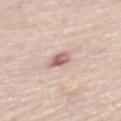Captured during whole-body skin photography for melanoma surveillance; the lesion was not biopsied. A 15 mm crop from a total-body photograph taken for skin-cancer surveillance. About 2.5 mm across. The tile uses white-light illumination. A male patient in their mid-80s or older. Located on the back.